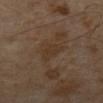Clinical impression:
Captured during whole-body skin photography for melanoma surveillance; the lesion was not biopsied.
Acquisition and patient details:
This is a cross-polarized tile. A male subject roughly 65 years of age. A 15 mm crop from a total-body photograph taken for skin-cancer surveillance. About 3 mm across.